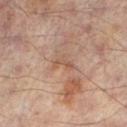<case>
<biopsy_status>not biopsied; imaged during a skin examination</biopsy_status>
<image>
  <source>total-body photography crop</source>
  <field_of_view_mm>15</field_of_view_mm>
</image>
<lighting>cross-polarized</lighting>
<lesion_size>
  <long_diameter_mm_approx>2.5</long_diameter_mm_approx>
</lesion_size>
<automated_metrics>
  <cielab_L>52</cielab_L>
  <cielab_a>19</cielab_a>
  <cielab_b>29</cielab_b>
  <vs_skin_contrast_norm>5.5</vs_skin_contrast_norm>
  <border_irregularity_0_10>6.5</border_irregularity_0_10>
  <color_variation_0_10>0.0</color_variation_0_10>
  <peripheral_color_asymmetry>0.0</peripheral_color_asymmetry>
  <nevus_likeness_0_100>0</nevus_likeness_0_100>
  <lesion_detection_confidence_0_100>100</lesion_detection_confidence_0_100>
</automated_metrics>
<site>left lower leg</site>
<patient>
  <sex>male</sex>
  <age_approx>65</age_approx>
</patient>
</case>